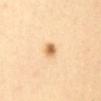About 2.5 mm across.
The subject is a female in their mid-40s.
Imaged with cross-polarized lighting.
A roughly 15 mm field-of-view crop from a total-body skin photograph.
The lesion is located on the abdomen.